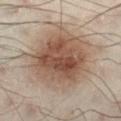{
  "biopsy_status": "not biopsied; imaged during a skin examination",
  "image": {
    "source": "total-body photography crop",
    "field_of_view_mm": 15
  },
  "patient": {
    "sex": "male",
    "age_approx": 50
  },
  "site": "left lower leg"
}